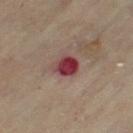| feature | finding |
|---|---|
| notes | no biopsy performed (imaged during a skin exam) |
| subject | female, aged approximately 60 |
| image | ~15 mm crop, total-body skin-cancer survey |
| location | the left thigh |